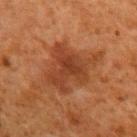Clinical summary:
A close-up tile cropped from a whole-body skin photograph, about 15 mm across. The recorded lesion diameter is about 6.5 mm. Automated tile analysis of the lesion measured a footprint of about 21 mm², a shape eccentricity near 0.65, and a symmetry-axis asymmetry near 0.45. It also reported a lesion color around L≈35 a*≈23 b*≈31 in CIELAB, about 8 CIELAB-L* units darker than the surrounding skin, and a lesion-to-skin contrast of about 7 (normalized; higher = more distinct). The analysis additionally found a within-lesion color-variation index near 3.5/10 and peripheral color asymmetry of about 1. Captured under cross-polarized illumination. From the left upper arm. A male subject roughly 60 years of age.